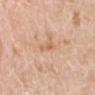Impression: The lesion was photographed on a routine skin check and not biopsied; there is no pathology result. Acquisition and patient details: Captured under white-light illumination. A 15 mm close-up extracted from a 3D total-body photography capture. A female subject approximately 70 years of age. Located on the arm. About 3 mm across.